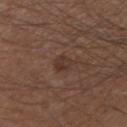Captured during whole-body skin photography for melanoma surveillance; the lesion was not biopsied. A male patient approximately 50 years of age. The lesion is on the arm. A close-up tile cropped from a whole-body skin photograph, about 15 mm across.